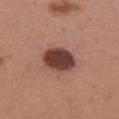Recorded during total-body skin imaging; not selected for excision or biopsy.
This image is a 15 mm lesion crop taken from a total-body photograph.
The lesion is on the chest.
This is a white-light tile.
The total-body-photography lesion software estimated an eccentricity of roughly 0.7. And it measured a mean CIELAB color near L≈40 a*≈22 b*≈23, roughly 18 lightness units darker than nearby skin, and a lesion-to-skin contrast of about 13 (normalized; higher = more distinct). The software also gave an automated nevus-likeness rating near 85 out of 100 and lesion-presence confidence of about 100/100.
The lesion's longest dimension is about 4.5 mm.
A female patient approximately 25 years of age.